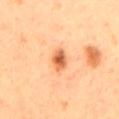The lesion was photographed on a routine skin check and not biopsied; there is no pathology result.
The lesion's longest dimension is about 3 mm.
A region of skin cropped from a whole-body photographic capture, roughly 15 mm wide.
A male subject aged 48 to 52.
The lesion is located on the mid back.